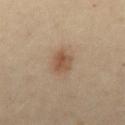The lesion was photographed on a routine skin check and not biopsied; there is no pathology result. This image is a 15 mm lesion crop taken from a total-body photograph. The recorded lesion diameter is about 2.5 mm. An algorithmic analysis of the crop reported a mean CIELAB color near L≈40 a*≈14 b*≈25, a lesion–skin lightness drop of about 8, and a lesion-to-skin contrast of about 7 (normalized; higher = more distinct). And it measured a border-irregularity index near 2/10, internal color variation of about 4 on a 0–10 scale, and a peripheral color-asymmetry measure near 1.5. On the abdomen. A female subject about 30 years old. Captured under cross-polarized illumination.